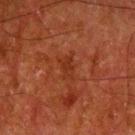This lesion was catalogued during total-body skin photography and was not selected for biopsy. An algorithmic analysis of the crop reported a mean CIELAB color near L≈26 a*≈24 b*≈29, roughly 5 lightness units darker than nearby skin, and a normalized border contrast of about 5.5. It also reported a border-irregularity index near 3.5/10, internal color variation of about 2 on a 0–10 scale, and peripheral color asymmetry of about 1. The analysis additionally found a classifier nevus-likeness of about 0/100 and a detector confidence of about 100 out of 100 that the crop contains a lesion. From the left upper arm. Cropped from a whole-body photographic skin survey; the tile spans about 15 mm. The lesion's longest dimension is about 3 mm. A male subject, approximately 80 years of age.